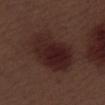Impression:
Imaged during a routine full-body skin examination; the lesion was not biopsied and no histopathology is available.
Background:
A region of skin cropped from a whole-body photographic capture, roughly 15 mm wide. Automated tile analysis of the lesion measured an area of roughly 26 mm², a shape eccentricity near 0.75, and two-axis asymmetry of about 0.15. It also reported a mean CIELAB color near L≈23 a*≈19 b*≈18, a lesion–skin lightness drop of about 8, and a normalized lesion–skin contrast near 9.5. And it measured a border-irregularity index near 2/10, internal color variation of about 5 on a 0–10 scale, and peripheral color asymmetry of about 1.5. And it measured an automated nevus-likeness rating near 90 out of 100 and lesion-presence confidence of about 100/100. From the leg. The recorded lesion diameter is about 7 mm. The patient is a male aged approximately 70.